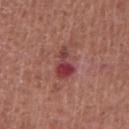<tbp_lesion>
  <biopsy_status>not biopsied; imaged during a skin examination</biopsy_status>
  <automated_metrics>
    <border_irregularity_0_10>5.5</border_irregularity_0_10>
    <color_variation_0_10>2.5</color_variation_0_10>
    <peripheral_color_asymmetry>0.5</peripheral_color_asymmetry>
  </automated_metrics>
  <patient>
    <sex>male</sex>
    <age_approx>65</age_approx>
  </patient>
  <lighting>white-light</lighting>
  <lesion_size>
    <long_diameter_mm_approx>4.0</long_diameter_mm_approx>
  </lesion_size>
  <image>
    <source>total-body photography crop</source>
    <field_of_view_mm>15</field_of_view_mm>
  </image>
  <site>front of the torso</site>
</tbp_lesion>